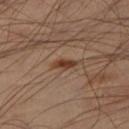{
  "biopsy_status": "not biopsied; imaged during a skin examination",
  "site": "right thigh",
  "lesion_size": {
    "long_diameter_mm_approx": 3.0
  },
  "lighting": "cross-polarized",
  "image": {
    "source": "total-body photography crop",
    "field_of_view_mm": 15
  },
  "patient": {
    "sex": "male",
    "age_approx": 55
  }
}